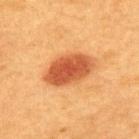Q: Was this lesion biopsied?
A: no biopsy performed (imaged during a skin exam)
Q: Illumination type?
A: cross-polarized illumination
Q: What are the patient's age and sex?
A: female, aged 38–42
Q: Lesion location?
A: the upper back
Q: What is the imaging modality?
A: ~15 mm tile from a whole-body skin photo